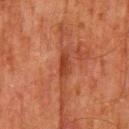This lesion was catalogued during total-body skin photography and was not selected for biopsy.
This is a cross-polarized tile.
Located on the back.
A male patient, in their 80s.
Longest diameter approximately 2.5 mm.
Cropped from a whole-body photographic skin survey; the tile spans about 15 mm.